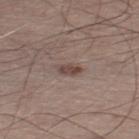Clinical impression: The lesion was photographed on a routine skin check and not biopsied; there is no pathology result. Acquisition and patient details: This image is a 15 mm lesion crop taken from a total-body photograph. The patient is a male aged 53 to 57. From the leg.